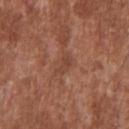Captured during whole-body skin photography for melanoma surveillance; the lesion was not biopsied.
This is a white-light tile.
On the upper back.
A male subject, in their mid-40s.
Approximately 3 mm at its widest.
A region of skin cropped from a whole-body photographic capture, roughly 15 mm wide.
The lesion-visualizer software estimated a lesion color around L≈43 a*≈23 b*≈28 in CIELAB, a lesion–skin lightness drop of about 7, and a normalized border contrast of about 5.5. The analysis additionally found a border-irregularity index near 5.5/10 and peripheral color asymmetry of about 0. And it measured an automated nevus-likeness rating near 0 out of 100 and lesion-presence confidence of about 85/100.